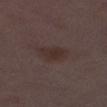Assessment:
This lesion was catalogued during total-body skin photography and was not selected for biopsy.
Background:
A lesion tile, about 15 mm wide, cut from a 3D total-body photograph. A female subject aged around 30. From the left thigh.